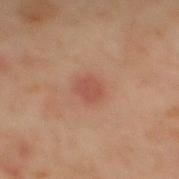This lesion was catalogued during total-body skin photography and was not selected for biopsy. About 2.5 mm across. On the mid back. This is a cross-polarized tile. The subject is a male aged 58–62. A lesion tile, about 15 mm wide, cut from a 3D total-body photograph. Automated image analysis of the tile measured a shape-asymmetry score of about 0.25 (0 = symmetric). And it measured a mean CIELAB color near L≈47 a*≈24 b*≈27, roughly 7 lightness units darker than nearby skin, and a normalized border contrast of about 5.5. It also reported a border-irregularity index near 2.5/10, internal color variation of about 1.5 on a 0–10 scale, and a peripheral color-asymmetry measure near 0.5.